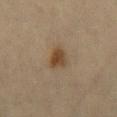The lesion was tiled from a total-body skin photograph and was not biopsied. A female subject, approximately 45 years of age. A lesion tile, about 15 mm wide, cut from a 3D total-body photograph. On the mid back.